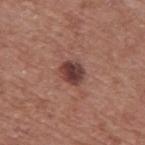Q: What is the lesion's diameter?
A: about 3 mm
Q: What is the imaging modality?
A: 15 mm crop, total-body photography
Q: What are the patient's age and sex?
A: male, roughly 75 years of age
Q: Illumination type?
A: white-light illumination
Q: Lesion location?
A: the mid back